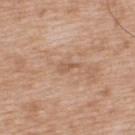biopsy status: total-body-photography surveillance lesion; no biopsy | lesion size: ~2.5 mm (longest diameter) | site: the upper back | lighting: white-light | subject: male, roughly 50 years of age | image source: ~15 mm tile from a whole-body skin photo.